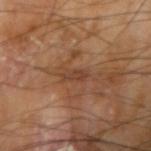Impression:
No biopsy was performed on this lesion — it was imaged during a full skin examination and was not determined to be concerning.
Context:
Cropped from a total-body skin-imaging series; the visible field is about 15 mm. Located on the right upper arm. The subject is a male aged 63–67.